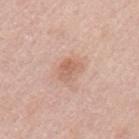notes: no biopsy performed (imaged during a skin exam)
size: ~3 mm (longest diameter)
image source: total-body-photography crop, ~15 mm field of view
subject: female, aged around 65
location: the left upper arm